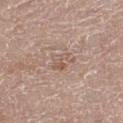Q: Is there a histopathology result?
A: imaged on a skin check; not biopsied
Q: What kind of image is this?
A: ~15 mm crop, total-body skin-cancer survey
Q: Who is the patient?
A: female, in their mid-60s
Q: Where on the body is the lesion?
A: the right lower leg